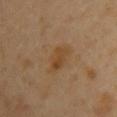Q: Was a biopsy performed?
A: imaged on a skin check; not biopsied
Q: What is the anatomic site?
A: the chest
Q: How large is the lesion?
A: ~4 mm (longest diameter)
Q: Patient demographics?
A: female, aged around 50
Q: What did automated image analysis measure?
A: an area of roughly 6 mm², an eccentricity of roughly 0.85, and a symmetry-axis asymmetry near 0.3; a mean CIELAB color near L≈43 a*≈17 b*≈34, a lesion–skin lightness drop of about 6, and a normalized border contrast of about 6.5
Q: What kind of image is this?
A: total-body-photography crop, ~15 mm field of view
Q: Illumination type?
A: cross-polarized illumination